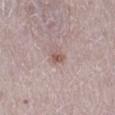This lesion was catalogued during total-body skin photography and was not selected for biopsy. The patient is a female aged around 50. The lesion's longest dimension is about 2 mm. A 15 mm close-up extracted from a 3D total-body photography capture. Captured under white-light illumination. The lesion is on the right lower leg.